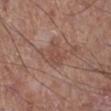Longest diameter approximately 3.5 mm.
On the right lower leg.
The tile uses white-light illumination.
A roughly 15 mm field-of-view crop from a total-body skin photograph.
An algorithmic analysis of the crop reported an area of roughly 7 mm², an outline eccentricity of about 0.35 (0 = round, 1 = elongated), and a shape-asymmetry score of about 0.3 (0 = symmetric). The analysis additionally found a border-irregularity rating of about 3/10, a color-variation rating of about 2/10, and a peripheral color-asymmetry measure near 0.5. The analysis additionally found a nevus-likeness score of about 0/100 and lesion-presence confidence of about 100/100.
A male subject roughly 65 years of age.